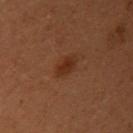Clinical impression: No biopsy was performed on this lesion — it was imaged during a full skin examination and was not determined to be concerning. Clinical summary: About 3 mm across. A female subject aged 48 to 52. Located on the right upper arm. The lesion-visualizer software estimated a lesion area of about 4 mm² and an eccentricity of roughly 0.8. It also reported a mean CIELAB color near L≈25 a*≈20 b*≈27 and a lesion–skin lightness drop of about 7. The analysis additionally found a classifier nevus-likeness of about 95/100 and lesion-presence confidence of about 100/100. This is a cross-polarized tile. Cropped from a total-body skin-imaging series; the visible field is about 15 mm.